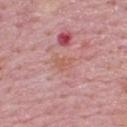notes: total-body-photography surveillance lesion; no biopsy | illumination: white-light illumination | image source: ~15 mm crop, total-body skin-cancer survey | diameter: about 2.5 mm | subject: male, in their mid-60s | body site: the back.